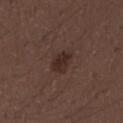Part of a total-body skin-imaging series; this lesion was reviewed on a skin check and was not flagged for biopsy. Imaged with white-light lighting. A male subject in their 30s. Automated tile analysis of the lesion measured a lesion color around L≈27 a*≈16 b*≈19 in CIELAB, roughly 8 lightness units darker than nearby skin, and a normalized lesion–skin contrast near 8.5. The software also gave border irregularity of about 3 on a 0–10 scale and a color-variation rating of about 2/10. It also reported an automated nevus-likeness rating near 70 out of 100. The lesion is on the left forearm. This image is a 15 mm lesion crop taken from a total-body photograph. Approximately 3.5 mm at its widest.